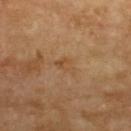{
  "biopsy_status": "not biopsied; imaged during a skin examination",
  "lesion_size": {
    "long_diameter_mm_approx": 2.5
  },
  "image": {
    "source": "total-body photography crop",
    "field_of_view_mm": 15
  },
  "automated_metrics": {
    "area_mm2_approx": 3.5,
    "shape_asymmetry": 0.35,
    "cielab_L": 50,
    "cielab_a": 20,
    "cielab_b": 36,
    "vs_skin_darker_L": 6.0,
    "vs_skin_contrast_norm": 5.0,
    "nevus_likeness_0_100": 0
  },
  "patient": {
    "sex": "female",
    "age_approx": 70
  },
  "site": "left forearm"
}